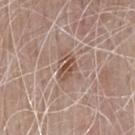biopsy status: imaged on a skin check; not biopsied.